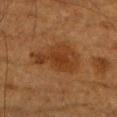biopsy status=catalogued during a skin exam; not biopsied | body site=the right upper arm | image source=total-body-photography crop, ~15 mm field of view | patient=male, aged around 85 or older.